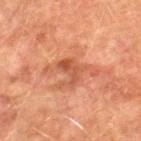The lesion was photographed on a routine skin check and not biopsied; there is no pathology result. A male subject aged around 75. Longest diameter approximately 3.5 mm. A 15 mm crop from a total-body photograph taken for skin-cancer surveillance. Imaged with cross-polarized lighting. The lesion is located on the right lower leg.